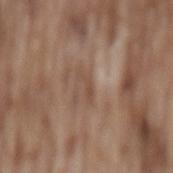Recorded during total-body skin imaging; not selected for excision or biopsy. A male subject, about 75 years old. A lesion tile, about 15 mm wide, cut from a 3D total-body photograph. The lesion is located on the back.